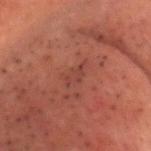{
  "biopsy_status": "not biopsied; imaged during a skin examination",
  "lesion_size": {
    "long_diameter_mm_approx": 3.0
  },
  "image": {
    "source": "total-body photography crop",
    "field_of_view_mm": 15
  },
  "patient": {
    "sex": "male",
    "age_approx": 50
  },
  "automated_metrics": {
    "area_mm2_approx": 3.0,
    "shape_asymmetry": 0.3,
    "cielab_L": 31,
    "cielab_a": 21,
    "cielab_b": 22,
    "vs_skin_darker_L": 5.0
  },
  "lighting": "cross-polarized",
  "site": "head or neck"
}